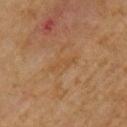Recorded during total-body skin imaging; not selected for excision or biopsy.
The subject is a female aged approximately 70.
This image is a 15 mm lesion crop taken from a total-body photograph.
From the arm.